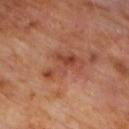Captured during whole-body skin photography for melanoma surveillance; the lesion was not biopsied.
The lesion's longest dimension is about 5.5 mm.
The lesion-visualizer software estimated an outline eccentricity of about 0.85 (0 = round, 1 = elongated) and a shape-asymmetry score of about 0.6 (0 = symmetric). It also reported a mean CIELAB color near L≈43 a*≈25 b*≈30, roughly 9 lightness units darker than nearby skin, and a normalized lesion–skin contrast near 7. It also reported border irregularity of about 8 on a 0–10 scale, a within-lesion color-variation index near 5/10, and a peripheral color-asymmetry measure near 1.5.
A male subject in their 60s.
Located on the upper back.
A lesion tile, about 15 mm wide, cut from a 3D total-body photograph.
Imaged with cross-polarized lighting.